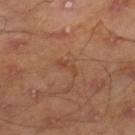Imaged during a routine full-body skin examination; the lesion was not biopsied and no histopathology is available. The lesion is located on the right lower leg. A male subject about 45 years old. Longest diameter approximately 2.5 mm. Cropped from a total-body skin-imaging series; the visible field is about 15 mm. The tile uses cross-polarized illumination.